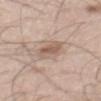Notes:
* workup — imaged on a skin check; not biopsied
* imaging modality — ~15 mm crop, total-body skin-cancer survey
* illumination — white-light
* lesion diameter — ≈5 mm
* anatomic site — the mid back
* subject — male, aged around 50